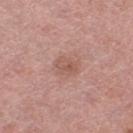Clinical impression:
The lesion was tiled from a total-body skin photograph and was not biopsied.
Clinical summary:
The patient is a female approximately 70 years of age. A 15 mm crop from a total-body photograph taken for skin-cancer surveillance. The lesion-visualizer software estimated an average lesion color of about L≈56 a*≈22 b*≈26 (CIELAB) and about 7 CIELAB-L* units darker than the surrounding skin. And it measured a classifier nevus-likeness of about 0/100 and lesion-presence confidence of about 100/100. This is a white-light tile. On the right thigh.